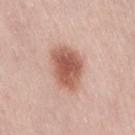{"biopsy_status": "not biopsied; imaged during a skin examination", "image": {"source": "total-body photography crop", "field_of_view_mm": 15}, "patient": {"sex": "female", "age_approx": 30}, "site": "lower back"}